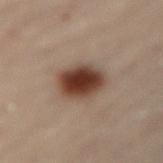Q: What are the patient's age and sex?
A: female, aged around 60
Q: Automated lesion metrics?
A: an area of roughly 12 mm² and a shape eccentricity near 0.4; a border-irregularity rating of about 1/10 and a color-variation rating of about 5/10
Q: Where on the body is the lesion?
A: the back
Q: What lighting was used for the tile?
A: cross-polarized illumination
Q: Lesion size?
A: ~4 mm (longest diameter)
Q: How was this image acquired?
A: total-body-photography crop, ~15 mm field of view